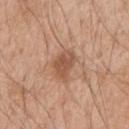Q: Is there a histopathology result?
A: catalogued during a skin exam; not biopsied
Q: Who is the patient?
A: male, approximately 50 years of age
Q: What kind of image is this?
A: 15 mm crop, total-body photography
Q: What is the anatomic site?
A: the left upper arm